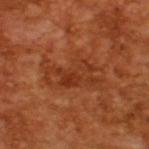{
  "biopsy_status": "not biopsied; imaged during a skin examination",
  "lighting": "cross-polarized",
  "image": {
    "source": "total-body photography crop",
    "field_of_view_mm": 15
  },
  "patient": {
    "sex": "male",
    "age_approx": 65
  },
  "automated_metrics": {
    "area_mm2_approx": 18.0,
    "eccentricity": 0.85,
    "shape_asymmetry": 0.4,
    "cielab_L": 37,
    "cielab_a": 28,
    "cielab_b": 36,
    "vs_skin_darker_L": 7.0,
    "vs_skin_contrast_norm": 6.0
  }
}